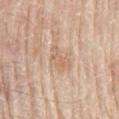Part of a total-body skin-imaging series; this lesion was reviewed on a skin check and was not flagged for biopsy.
The lesion-visualizer software estimated an eccentricity of roughly 0.85 and a shape-asymmetry score of about 0.45 (0 = symmetric). And it measured a border-irregularity rating of about 5/10, a color-variation rating of about 1/10, and peripheral color asymmetry of about 0.5. The analysis additionally found a detector confidence of about 100 out of 100 that the crop contains a lesion.
A male subject in their 80s.
A roughly 15 mm field-of-view crop from a total-body skin photograph.
Captured under white-light illumination.
Located on the mid back.